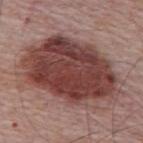<case>
<biopsy_status>not biopsied; imaged during a skin examination</biopsy_status>
<site>upper back</site>
<lighting>white-light</lighting>
<patient>
  <sex>male</sex>
  <age_approx>65</age_approx>
</patient>
<image>
  <source>total-body photography crop</source>
  <field_of_view_mm>15</field_of_view_mm>
</image>
<automated_metrics>
  <area_mm2_approx>60.0</area_mm2_approx>
  <eccentricity>0.8</eccentricity>
  <cielab_L>41</cielab_L>
  <cielab_a>23</cielab_a>
  <cielab_b>22</cielab_b>
  <vs_skin_darker_L>16.0</vs_skin_darker_L>
  <vs_skin_contrast_norm>11.5</vs_skin_contrast_norm>
  <color_variation_0_10>6.0</color_variation_0_10>
  <peripheral_color_asymmetry>2.0</peripheral_color_asymmetry>
  <lesion_detection_confidence_0_100>100</lesion_detection_confidence_0_100>
</automated_metrics>
</case>